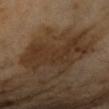biopsy status = total-body-photography surveillance lesion; no biopsy | subject = female, in their 70s | image source = ~15 mm tile from a whole-body skin photo | location = the right forearm.